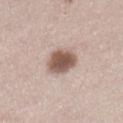| field | value |
|---|---|
| follow-up | imaged on a skin check; not biopsied |
| image-analysis metrics | a lesion area of about 10 mm² and an outline eccentricity of about 0.6 (0 = round, 1 = elongated); a nevus-likeness score of about 95/100 and lesion-presence confidence of about 100/100 |
| acquisition | 15 mm crop, total-body photography |
| size | ~4 mm (longest diameter) |
| illumination | white-light |
| location | the right lower leg |
| patient | female, aged 38–42 |